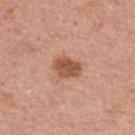workup: no biopsy performed (imaged during a skin exam)
acquisition: ~15 mm tile from a whole-body skin photo
lighting: white-light illumination
image-analysis metrics: a shape eccentricity near 0.65 and a symmetry-axis asymmetry near 0.2; a lesion color around L≈53 a*≈24 b*≈31 in CIELAB, a lesion–skin lightness drop of about 13, and a normalized lesion–skin contrast near 9; a border-irregularity index near 2/10, internal color variation of about 2.5 on a 0–10 scale, and peripheral color asymmetry of about 1
patient: female, aged 33 to 37
anatomic site: the left forearm